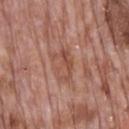{
  "biopsy_status": "not biopsied; imaged during a skin examination"
}